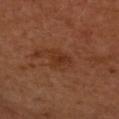Q: Is there a histopathology result?
A: imaged on a skin check; not biopsied
Q: Automated lesion metrics?
A: a color-variation rating of about 2.5/10 and a peripheral color-asymmetry measure near 1; a nevus-likeness score of about 0/100 and lesion-presence confidence of about 100/100
Q: Illumination type?
A: cross-polarized illumination
Q: What is the lesion's diameter?
A: ≈3 mm
Q: Where on the body is the lesion?
A: the left upper arm
Q: How was this image acquired?
A: ~15 mm crop, total-body skin-cancer survey
Q: Patient demographics?
A: female, approximately 65 years of age